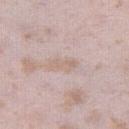No biopsy was performed on this lesion — it was imaged during a full skin examination and was not determined to be concerning.
A 15 mm crop from a total-body photograph taken for skin-cancer surveillance.
Measured at roughly 3.5 mm in maximum diameter.
The tile uses white-light illumination.
An algorithmic analysis of the crop reported a lesion color around L≈65 a*≈15 b*≈24 in CIELAB, roughly 6 lightness units darker than nearby skin, and a normalized border contrast of about 5.
On the right thigh.
A female subject, aged approximately 25.